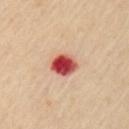Captured during whole-body skin photography for melanoma surveillance; the lesion was not biopsied.
A male subject aged approximately 55.
An algorithmic analysis of the crop reported a mean CIELAB color near L≈53 a*≈38 b*≈31, about 22 CIELAB-L* units darker than the surrounding skin, and a normalized lesion–skin contrast near 13.5. And it measured an automated nevus-likeness rating near 0 out of 100.
On the left upper arm.
Cropped from a total-body skin-imaging series; the visible field is about 15 mm.
The recorded lesion diameter is about 3 mm.
This is a cross-polarized tile.